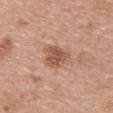biopsy status: imaged on a skin check; not biopsied
tile lighting: white-light illumination
image-analysis metrics: a lesion area of about 9 mm² and a shape-asymmetry score of about 0.3 (0 = symmetric); a nevus-likeness score of about 75/100
subject: female, aged approximately 60
acquisition: 15 mm crop, total-body photography
anatomic site: the left upper arm
lesion size: about 4.5 mm